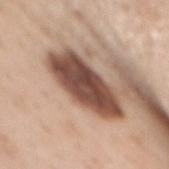follow-up: total-body-photography surveillance lesion; no biopsy | illumination: white-light | automated metrics: a lesion color around L≈47 a*≈20 b*≈26 in CIELAB, a lesion–skin lightness drop of about 21, and a lesion-to-skin contrast of about 14 (normalized; higher = more distinct); border irregularity of about 3 on a 0–10 scale, a within-lesion color-variation index near 5/10, and a peripheral color-asymmetry measure near 1.5; a detector confidence of about 100 out of 100 that the crop contains a lesion | subject: female, approximately 50 years of age | image source: ~15 mm tile from a whole-body skin photo | diameter: ~9 mm (longest diameter) | site: the back.